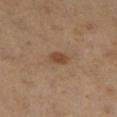The lesion was photographed on a routine skin check and not biopsied; there is no pathology result.